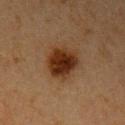{"biopsy_status": "not biopsied; imaged during a skin examination", "patient": {"sex": "female", "age_approx": 60}, "site": "arm", "image": {"source": "total-body photography crop", "field_of_view_mm": 15}}